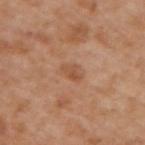A roughly 15 mm field-of-view crop from a total-body skin photograph.
The lesion's longest dimension is about 3 mm.
A male subject aged around 65.
The lesion is on the upper back.
The tile uses white-light illumination.